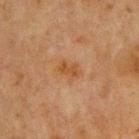This lesion was catalogued during total-body skin photography and was not selected for biopsy.
A roughly 15 mm field-of-view crop from a total-body skin photograph.
Measured at roughly 2.5 mm in maximum diameter.
A male subject in their mid-60s.
An algorithmic analysis of the crop reported a lesion color around L≈40 a*≈20 b*≈33 in CIELAB, roughly 6 lightness units darker than nearby skin, and a normalized lesion–skin contrast near 7. The analysis additionally found border irregularity of about 2.5 on a 0–10 scale, internal color variation of about 1 on a 0–10 scale, and radial color variation of about 0.5. The software also gave a classifier nevus-likeness of about 5/100.
Imaged with cross-polarized lighting.
From the chest.